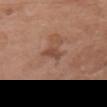Captured during whole-body skin photography for melanoma surveillance; the lesion was not biopsied. Imaged with white-light lighting. The lesion's longest dimension is about 2.5 mm. A female subject, in their mid-70s. The lesion is located on the front of the torso. This image is a 15 mm lesion crop taken from a total-body photograph. The total-body-photography lesion software estimated a footprint of about 3 mm², a shape eccentricity near 0.8, and a shape-asymmetry score of about 0.4 (0 = symmetric). It also reported about 8 CIELAB-L* units darker than the surrounding skin and a normalized border contrast of about 6.5.